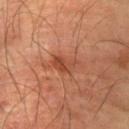biopsy status: no biopsy performed (imaged during a skin exam) | body site: the left arm | image-analysis metrics: a shape eccentricity near 0.75 and a shape-asymmetry score of about 0.35 (0 = symmetric); a lesion color around L≈45 a*≈27 b*≈33 in CIELAB, about 9 CIELAB-L* units darker than the surrounding skin, and a lesion-to-skin contrast of about 6.5 (normalized; higher = more distinct) | imaging modality: total-body-photography crop, ~15 mm field of view | patient: male, aged 58 to 62 | size: ~3 mm (longest diameter) | illumination: cross-polarized.